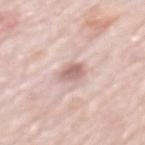Q: Is there a histopathology result?
A: no biopsy performed (imaged during a skin exam)
Q: Illumination type?
A: white-light
Q: Lesion location?
A: the mid back
Q: How was this image acquired?
A: ~15 mm crop, total-body skin-cancer survey
Q: Patient demographics?
A: male, aged approximately 80